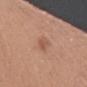Impression: Captured during whole-body skin photography for melanoma surveillance; the lesion was not biopsied. Clinical summary: Located on the upper back. A lesion tile, about 15 mm wide, cut from a 3D total-body photograph. A female subject, aged around 70. The recorded lesion diameter is about 3 mm. Captured under white-light illumination.